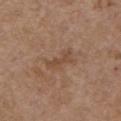<record>
  <biopsy_status>not biopsied; imaged during a skin examination</biopsy_status>
  <patient>
    <sex>female</sex>
    <age_approx>65</age_approx>
  </patient>
  <image>
    <source>total-body photography crop</source>
    <field_of_view_mm>15</field_of_view_mm>
  </image>
  <site>front of the torso</site>
  <lighting>white-light</lighting>
</record>